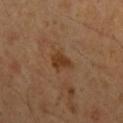Findings:
• illumination · cross-polarized illumination
• anatomic site · the left upper arm
• acquisition · total-body-photography crop, ~15 mm field of view
• patient · male, in their mid-40s
• automated metrics · a lesion area of about 4.5 mm² and a shape eccentricity near 0.7; a normalized lesion–skin contrast near 8.5; a border-irregularity index near 3/10, internal color variation of about 1.5 on a 0–10 scale, and radial color variation of about 0.5; a classifier nevus-likeness of about 65/100 and a detector confidence of about 100 out of 100 that the crop contains a lesion